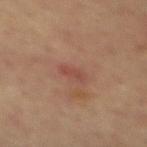Q: Was a biopsy performed?
A: imaged on a skin check; not biopsied
Q: Where on the body is the lesion?
A: the mid back
Q: What lighting was used for the tile?
A: cross-polarized illumination
Q: What is the lesion's diameter?
A: about 2.5 mm
Q: What are the patient's age and sex?
A: male, in their mid-60s
Q: How was this image acquired?
A: ~15 mm crop, total-body skin-cancer survey